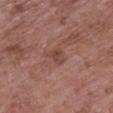Case summary:
– workup — catalogued during a skin exam; not biopsied
– body site — the right upper arm
– acquisition — 15 mm crop, total-body photography
– tile lighting — white-light illumination
– subject — female, roughly 70 years of age
– image-analysis metrics — an average lesion color of about L≈44 a*≈23 b*≈24 (CIELAB), a lesion–skin lightness drop of about 7, and a normalized lesion–skin contrast near 6; radial color variation of about 1; a lesion-detection confidence of about 100/100